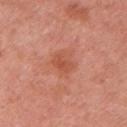The lesion was photographed on a routine skin check and not biopsied; there is no pathology result. From the left upper arm. This image is a 15 mm lesion crop taken from a total-body photograph. A female subject, aged around 50.